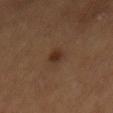Impression:
Part of a total-body skin-imaging series; this lesion was reviewed on a skin check and was not flagged for biopsy.
Image and clinical context:
A lesion tile, about 15 mm wide, cut from a 3D total-body photograph. The subject is a male aged approximately 55. Captured under cross-polarized illumination. Located on the mid back. About 2.5 mm across.